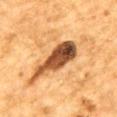Clinical impression:
The lesion was photographed on a routine skin check and not biopsied; there is no pathology result.
Acquisition and patient details:
A male patient, aged approximately 85 or older. About 7 mm across. Cropped from a whole-body photographic skin survey; the tile spans about 15 mm. On the back. Captured under cross-polarized illumination.